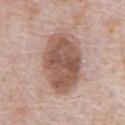Assessment: Imaged during a routine full-body skin examination; the lesion was not biopsied and no histopathology is available. Image and clinical context: The tile uses white-light illumination. A male subject roughly 70 years of age. The lesion-visualizer software estimated a lesion color around L≈54 a*≈19 b*≈26 in CIELAB, roughly 14 lightness units darker than nearby skin, and a normalized lesion–skin contrast near 9.5. From the chest. About 8 mm across. This image is a 15 mm lesion crop taken from a total-body photograph.